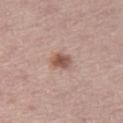Impression:
This lesion was catalogued during total-body skin photography and was not selected for biopsy.
Image and clinical context:
A female patient, about 55 years old. Approximately 2.5 mm at its widest. Captured under white-light illumination. A 15 mm close-up extracted from a 3D total-body photography capture. The lesion is on the left thigh.